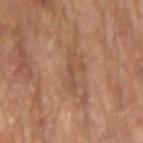  biopsy_status: not biopsied; imaged during a skin examination
  lesion_size:
    long_diameter_mm_approx: 3.5
  lighting: cross-polarized
  patient:
    sex: male
    age_approx: 65
  image:
    source: total-body photography crop
    field_of_view_mm: 15
  automated_metrics:
    area_mm2_approx: 2.5
    cielab_L: 48
    cielab_a: 18
    cielab_b: 29
    border_irregularity_0_10: 6.5
    lesion_detection_confidence_0_100: 85
  site: left forearm